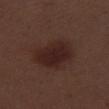The lesion was tiled from a total-body skin photograph and was not biopsied. The lesion is located on the right thigh. Captured under white-light illumination. A male patient, about 70 years old. The total-body-photography lesion software estimated a lesion color around L≈23 a*≈18 b*≈19 in CIELAB and about 8 CIELAB-L* units darker than the surrounding skin. And it measured a nevus-likeness score of about 90/100 and a detector confidence of about 100 out of 100 that the crop contains a lesion. A 15 mm close-up tile from a total-body photography series done for melanoma screening.